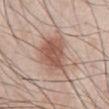biopsy status: imaged on a skin check; not biopsied | image-analysis metrics: a mean CIELAB color near L≈57 a*≈19 b*≈26, roughly 11 lightness units darker than nearby skin, and a lesion-to-skin contrast of about 7.5 (normalized; higher = more distinct); a nevus-likeness score of about 90/100 and a detector confidence of about 100 out of 100 that the crop contains a lesion | acquisition: ~15 mm tile from a whole-body skin photo | subject: male, aged approximately 60 | body site: the abdomen.